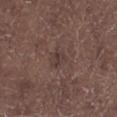follow-up: no biopsy performed (imaged during a skin exam) | image source: ~15 mm tile from a whole-body skin photo | patient: male, aged 68–72 | site: the left lower leg | automated lesion analysis: an eccentricity of roughly 0.6 and a symmetry-axis asymmetry near 0.3; a border-irregularity rating of about 2.5/10 and a peripheral color-asymmetry measure near 1 | lesion diameter: ~2.5 mm (longest diameter).